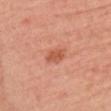The lesion was tiled from a total-body skin photograph and was not biopsied. The lesion is on the head or neck. A 15 mm close-up tile from a total-body photography series done for melanoma screening. A female subject, in their mid-70s. Longest diameter approximately 3 mm. This is a white-light tile.